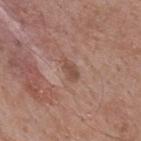Findings:
• biopsy status · no biopsy performed (imaged during a skin exam)